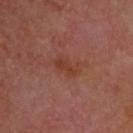Notes:
- follow-up — no biopsy performed (imaged during a skin exam)
- lesion size — ≈3 mm
- subject — male, aged around 65
- tile lighting — cross-polarized
- image — ~15 mm tile from a whole-body skin photo
- location — the head or neck
- automated metrics — a lesion color around L≈39 a*≈25 b*≈29 in CIELAB and roughly 6 lightness units darker than nearby skin; a detector confidence of about 100 out of 100 that the crop contains a lesion